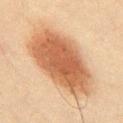Captured during whole-body skin photography for melanoma surveillance; the lesion was not biopsied.
The subject is a male aged 58 to 62.
A close-up tile cropped from a whole-body skin photograph, about 15 mm across.
Measured at roughly 9 mm in maximum diameter.
From the front of the torso.